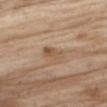follow-up = no biopsy performed (imaged during a skin exam); patient = male, aged 68–72; acquisition = 15 mm crop, total-body photography; body site = the right thigh; lighting = white-light illumination.